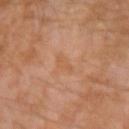notes = no biopsy performed (imaged during a skin exam) | diameter = about 3 mm | patient = male, approximately 30 years of age | acquisition = 15 mm crop, total-body photography | TBP lesion metrics = roughly 5 lightness units darker than nearby skin and a normalized border contrast of about 4.5 | lighting = cross-polarized illumination | site = the left arm.